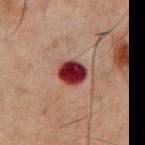notes: no biopsy performed (imaged during a skin exam) | image-analysis metrics: a footprint of about 7 mm² and a shape eccentricity near 0.3; a lesion-detection confidence of about 100/100 | tile lighting: cross-polarized illumination | patient: male, in their 60s | lesion size: ≈3 mm | image: 15 mm crop, total-body photography | body site: the chest.